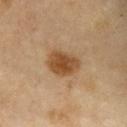<record>
<biopsy_status>not biopsied; imaged during a skin examination</biopsy_status>
<automated_metrics>
  <vs_skin_darker_L>12.0</vs_skin_darker_L>
  <nevus_likeness_0_100>100</nevus_likeness_0_100>
</automated_metrics>
<lesion_size>
  <long_diameter_mm_approx>4.0</long_diameter_mm_approx>
</lesion_size>
<patient>
  <sex>female</sex>
  <age_approx>60</age_approx>
</patient>
<lighting>cross-polarized</lighting>
<site>chest</site>
<image>
  <source>total-body photography crop</source>
  <field_of_view_mm>15</field_of_view_mm>
</image>
</record>